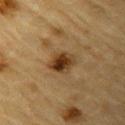Impression:
The lesion was photographed on a routine skin check and not biopsied; there is no pathology result.
Clinical summary:
On the left upper arm. A male patient, about 85 years old or older. Captured under cross-polarized illumination. A close-up tile cropped from a whole-body skin photograph, about 15 mm across. The lesion's longest dimension is about 3.5 mm.